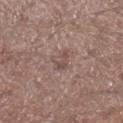Acquisition and patient details:
On the leg. The lesion-visualizer software estimated a mean CIELAB color near L≈49 a*≈17 b*≈21, a lesion–skin lightness drop of about 7, and a lesion-to-skin contrast of about 5.5 (normalized; higher = more distinct). And it measured a color-variation rating of about 3.5/10 and radial color variation of about 1.5. Measured at roughly 2.5 mm in maximum diameter. A male subject, about 55 years old. A region of skin cropped from a whole-body photographic capture, roughly 15 mm wide. Captured under white-light illumination.